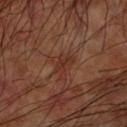Clinical summary:
Located on the left upper arm. The subject is a male about 60 years old. The tile uses cross-polarized illumination. Approximately 3 mm at its widest. A region of skin cropped from a whole-body photographic capture, roughly 15 mm wide. An algorithmic analysis of the crop reported a footprint of about 4 mm² and a symmetry-axis asymmetry near 0.4. The analysis additionally found an average lesion color of about L≈31 a*≈22 b*≈26 (CIELAB), a lesion–skin lightness drop of about 6, and a lesion-to-skin contrast of about 6 (normalized; higher = more distinct). The software also gave a border-irregularity index near 4/10, a within-lesion color-variation index near 1.5/10, and peripheral color asymmetry of about 0.5. And it measured a lesion-detection confidence of about 95/100.